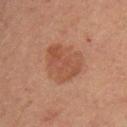Notes:
– subject · female, aged approximately 55
– tile lighting · cross-polarized
– image-analysis metrics · an area of roughly 18 mm², a shape eccentricity near 0.35, and a shape-asymmetry score of about 0.25 (0 = symmetric); a mean CIELAB color near L≈44 a*≈20 b*≈28, about 7 CIELAB-L* units darker than the surrounding skin, and a lesion-to-skin contrast of about 6 (normalized; higher = more distinct); border irregularity of about 2.5 on a 0–10 scale, a color-variation rating of about 3/10, and peripheral color asymmetry of about 1; a nevus-likeness score of about 40/100 and a detector confidence of about 100 out of 100 that the crop contains a lesion
– image · ~15 mm crop, total-body skin-cancer survey
– location · the right thigh